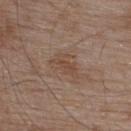The lesion was tiled from a total-body skin photograph and was not biopsied. Automated tile analysis of the lesion measured a border-irregularity index near 6/10, internal color variation of about 1.5 on a 0–10 scale, and radial color variation of about 0.5. The analysis additionally found an automated nevus-likeness rating near 0 out of 100. This image is a 15 mm lesion crop taken from a total-body photograph. The subject is a male approximately 55 years of age. From the upper back. Longest diameter approximately 4 mm. Imaged with white-light lighting.